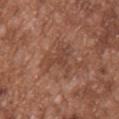workup: no biopsy performed (imaged during a skin exam) | site: the chest | patient: male, approximately 45 years of age | image source: 15 mm crop, total-body photography.